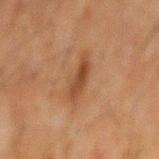Findings:
– biopsy status — total-body-photography surveillance lesion; no biopsy
– body site — the mid back
– lighting — cross-polarized illumination
– size — about 4.5 mm
– imaging modality — total-body-photography crop, ~15 mm field of view
– subject — male, in their 60s